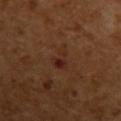Impression: No biopsy was performed on this lesion — it was imaged during a full skin examination and was not determined to be concerning.